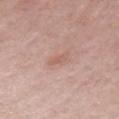Case summary:
- body site: the left upper arm
- image-analysis metrics: a footprint of about 3 mm², an outline eccentricity of about 0.8 (0 = round, 1 = elongated), and two-axis asymmetry of about 0.35; a border-irregularity rating of about 4/10
- image: ~15 mm tile from a whole-body skin photo
- subject: male, about 70 years old